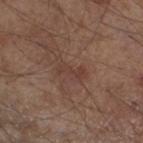follow-up=no biopsy performed (imaged during a skin exam)
tile lighting=white-light
patient=male, aged 53–57
image source=total-body-photography crop, ~15 mm field of view
location=the leg
lesion size=~3 mm (longest diameter)
automated metrics=border irregularity of about 6 on a 0–10 scale and a color-variation rating of about 0/10; a classifier nevus-likeness of about 0/100 and a lesion-detection confidence of about 100/100